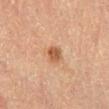biopsy_status: not biopsied; imaged during a skin examination
image:
  source: total-body photography crop
  field_of_view_mm: 15
lesion_size:
  long_diameter_mm_approx: 2.5
lighting: cross-polarized
site: left lower leg
patient:
  sex: male
  age_approx: 85+